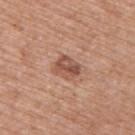Recorded during total-body skin imaging; not selected for excision or biopsy. A male subject aged 78 to 82. A roughly 15 mm field-of-view crop from a total-body skin photograph. From the back. An algorithmic analysis of the crop reported an area of roughly 6 mm² and an outline eccentricity of about 0.65 (0 = round, 1 = elongated).